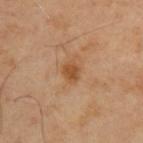Notes:
- notes — catalogued during a skin exam; not biopsied
- site — the back
- size — about 2.5 mm
- patient — male, about 40 years old
- image — total-body-photography crop, ~15 mm field of view